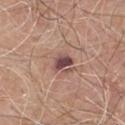biopsy_status: not biopsied; imaged during a skin examination
lighting: white-light
lesion_size:
  long_diameter_mm_approx: 3.0
site: chest
image:
  source: total-body photography crop
  field_of_view_mm: 15
patient:
  sex: male
  age_approx: 80